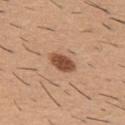{"biopsy_status": "not biopsied; imaged during a skin examination", "patient": {"sex": "male", "age_approx": 60}, "site": "upper back", "lighting": "white-light", "lesion_size": {"long_diameter_mm_approx": 3.5}, "image": {"source": "total-body photography crop", "field_of_view_mm": 15}}